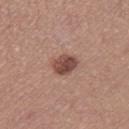follow-up: catalogued during a skin exam; not biopsied | patient: female, in their mid-30s | image: total-body-photography crop, ~15 mm field of view | automated metrics: a lesion area of about 6 mm², an eccentricity of roughly 0.55, and a shape-asymmetry score of about 0.2 (0 = symmetric); roughly 13 lightness units darker than nearby skin and a lesion-to-skin contrast of about 9.5 (normalized; higher = more distinct); an automated nevus-likeness rating near 55 out of 100 | size: ≈3 mm | location: the right thigh | lighting: white-light illumination.